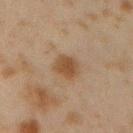Captured during whole-body skin photography for melanoma surveillance; the lesion was not biopsied. Automated tile analysis of the lesion measured an area of roughly 6 mm² and a shape-asymmetry score of about 0.2 (0 = symmetric). And it measured a mean CIELAB color near L≈39 a*≈15 b*≈28, about 8 CIELAB-L* units darker than the surrounding skin, and a lesion-to-skin contrast of about 8.5 (normalized; higher = more distinct). And it measured a border-irregularity rating of about 2/10, a within-lesion color-variation index near 1.5/10, and peripheral color asymmetry of about 0.5. A male patient aged approximately 45. On the arm. The lesion's longest dimension is about 3 mm. The tile uses cross-polarized illumination. This image is a 15 mm lesion crop taken from a total-body photograph.